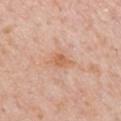No biopsy was performed on this lesion — it was imaged during a full skin examination and was not determined to be concerning. Captured under white-light illumination. The lesion is located on the mid back. A male patient aged around 60. The lesion-visualizer software estimated an area of roughly 4 mm² and a symmetry-axis asymmetry near 0.3. And it measured about 8 CIELAB-L* units darker than the surrounding skin and a normalized border contrast of about 7. And it measured a nevus-likeness score of about 0/100 and lesion-presence confidence of about 100/100. Longest diameter approximately 3 mm. A close-up tile cropped from a whole-body skin photograph, about 15 mm across.